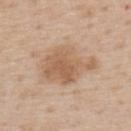| feature | finding |
|---|---|
| workup | total-body-photography surveillance lesion; no biopsy |
| anatomic site | the upper back |
| lighting | white-light illumination |
| automated metrics | a lesion area of about 20 mm², an eccentricity of roughly 0.75, and two-axis asymmetry of about 0.3; a lesion color around L≈60 a*≈18 b*≈32 in CIELAB, a lesion–skin lightness drop of about 10, and a normalized lesion–skin contrast near 7; a classifier nevus-likeness of about 10/100 and a lesion-detection confidence of about 100/100 |
| patient | male, aged approximately 60 |
| image | ~15 mm crop, total-body skin-cancer survey |